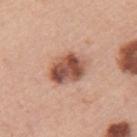Clinical summary:
Located on the left upper arm. A female subject roughly 60 years of age. Cropped from a whole-body photographic skin survey; the tile spans about 15 mm. Approximately 4.5 mm at its widest.